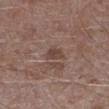{
  "biopsy_status": "not biopsied; imaged during a skin examination",
  "patient": {
    "sex": "male",
    "age_approx": 45
  },
  "image": {
    "source": "total-body photography crop",
    "field_of_view_mm": 15
  },
  "lesion_size": {
    "long_diameter_mm_approx": 3.0
  },
  "lighting": "white-light",
  "automated_metrics": {
    "cielab_L": 43,
    "cielab_a": 16,
    "cielab_b": 22,
    "vs_skin_darker_L": 8.0,
    "vs_skin_contrast_norm": 6.5
  },
  "site": "left lower leg"
}